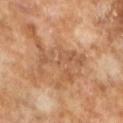Captured during whole-body skin photography for melanoma surveillance; the lesion was not biopsied. Cropped from a whole-body photographic skin survey; the tile spans about 15 mm. Captured under cross-polarized illumination. Approximately 6.5 mm at its widest. The subject is a male about 65 years old.